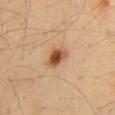follow-up: catalogued during a skin exam; not biopsied | anatomic site: the mid back | lesion size: about 3 mm | patient: male, aged 33 to 37 | image: ~15 mm tile from a whole-body skin photo.